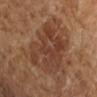Imaged during a routine full-body skin examination; the lesion was not biopsied and no histopathology is available.
About 9 mm across.
Imaged with cross-polarized lighting.
A lesion tile, about 15 mm wide, cut from a 3D total-body photograph.
A female subject approximately 70 years of age.
Located on the right upper arm.
Automated tile analysis of the lesion measured a footprint of about 36 mm², an outline eccentricity of about 0.8 (0 = round, 1 = elongated), and two-axis asymmetry of about 0.3. And it measured a nevus-likeness score of about 5/100.